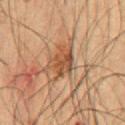Longest diameter approximately 3.5 mm. On the abdomen. This image is a 15 mm lesion crop taken from a total-body photograph. This is a cross-polarized tile. Automated tile analysis of the lesion measured a lesion area of about 7.5 mm² and an outline eccentricity of about 0.4 (0 = round, 1 = elongated). It also reported a nevus-likeness score of about 60/100 and a detector confidence of about 95 out of 100 that the crop contains a lesion. A male subject, approximately 50 years of age.